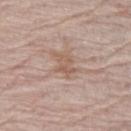Case summary:
• biopsy status — no biopsy performed (imaged during a skin exam)
• subject — female, about 65 years old
• lesion size — ≈3 mm
• image — 15 mm crop, total-body photography
• site — the left thigh
• automated metrics — a footprint of about 4.5 mm², an outline eccentricity of about 0.75 (0 = round, 1 = elongated), and a symmetry-axis asymmetry near 0.35; about 7 CIELAB-L* units darker than the surrounding skin and a normalized border contrast of about 5.5; border irregularity of about 4 on a 0–10 scale, a within-lesion color-variation index near 3/10, and peripheral color asymmetry of about 1; lesion-presence confidence of about 95/100
• illumination — white-light